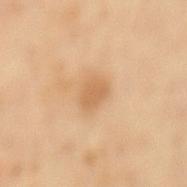Q: Was a biopsy performed?
A: catalogued during a skin exam; not biopsied
Q: Lesion location?
A: the mid back
Q: What lighting was used for the tile?
A: cross-polarized illumination
Q: What is the lesion's diameter?
A: ≈2.5 mm
Q: Who is the patient?
A: female, in their mid-60s
Q: What kind of image is this?
A: total-body-photography crop, ~15 mm field of view